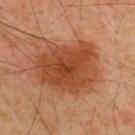Q: Is there a histopathology result?
A: imaged on a skin check; not biopsied
Q: What are the patient's age and sex?
A: male, aged 48–52
Q: Where on the body is the lesion?
A: the upper back
Q: What is the lesion's diameter?
A: ≈7.5 mm
Q: What kind of image is this?
A: ~15 mm tile from a whole-body skin photo
Q: How was the tile lit?
A: cross-polarized illumination